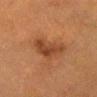follow-up: total-body-photography surveillance lesion; no biopsy | image: ~15 mm tile from a whole-body skin photo | site: the head or neck | size: ~4.5 mm (longest diameter) | patient: female, in their mid- to late 50s | tile lighting: cross-polarized.